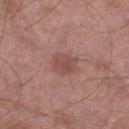| key | value |
|---|---|
| follow-up | catalogued during a skin exam; not biopsied |
| lesion size | ≈3 mm |
| image | 15 mm crop, total-body photography |
| site | the leg |
| patient | male, roughly 55 years of age |